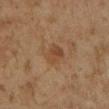The lesion was tiled from a total-body skin photograph and was not biopsied. Located on the left lower leg. This image is a 15 mm lesion crop taken from a total-body photograph. A male subject aged 58–62.